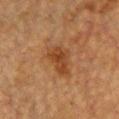The lesion was tiled from a total-body skin photograph and was not biopsied.
A female patient aged 53–57.
Located on the chest.
A close-up tile cropped from a whole-body skin photograph, about 15 mm across.
The recorded lesion diameter is about 4 mm.
The tile uses cross-polarized illumination.